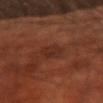Captured during whole-body skin photography for melanoma surveillance; the lesion was not biopsied. Measured at roughly 3 mm in maximum diameter. The lesion is on the front of the torso. This image is a 15 mm lesion crop taken from a total-body photograph. The subject is a male aged 68–72. This is a cross-polarized tile.